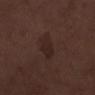{"lesion_size": {"long_diameter_mm_approx": 3.5}, "automated_metrics": {"border_irregularity_0_10": 2.5, "color_variation_0_10": 2.0, "peripheral_color_asymmetry": 0.5}, "patient": {"sex": "male", "age_approx": 70}, "site": "leg", "image": {"source": "total-body photography crop", "field_of_view_mm": 15}, "lighting": "white-light"}